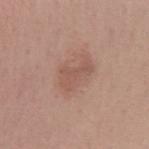Notes:
– workup · catalogued during a skin exam; not biopsied
– patient · female, in their 30s
– anatomic site · the right forearm
– size · about 4 mm
– tile lighting · white-light illumination
– image · 15 mm crop, total-body photography